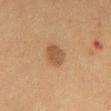notes = total-body-photography surveillance lesion; no biopsy
patient = female, approximately 50 years of age
body site = the chest
image source = 15 mm crop, total-body photography
lesion diameter = ~3 mm (longest diameter)